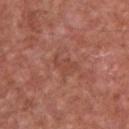Part of a total-body skin-imaging series; this lesion was reviewed on a skin check and was not flagged for biopsy. A male subject aged 63 to 67. Cropped from a total-body skin-imaging series; the visible field is about 15 mm. The lesion is located on the chest.